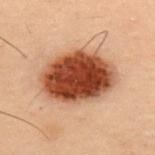Q: Was this lesion biopsied?
A: total-body-photography surveillance lesion; no biopsy
Q: Who is the patient?
A: male, aged 53 to 57
Q: What is the lesion's diameter?
A: about 8 mm
Q: Automated lesion metrics?
A: an area of roughly 35 mm², an eccentricity of roughly 0.7, and two-axis asymmetry of about 0.1; a mean CIELAB color near L≈39 a*≈23 b*≈29, about 19 CIELAB-L* units darker than the surrounding skin, and a normalized lesion–skin contrast near 14.5; internal color variation of about 7 on a 0–10 scale and radial color variation of about 2
Q: Illumination type?
A: cross-polarized
Q: What is the anatomic site?
A: the upper back
Q: What is the imaging modality?
A: 15 mm crop, total-body photography